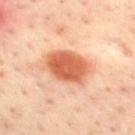The lesion was tiled from a total-body skin photograph and was not biopsied. The lesion is located on the upper back. Captured under cross-polarized illumination. Longest diameter approximately 5 mm. A male subject, approximately 45 years of age. Cropped from a total-body skin-imaging series; the visible field is about 15 mm.